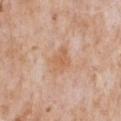Q: Was a biopsy performed?
A: no biopsy performed (imaged during a skin exam)
Q: Where on the body is the lesion?
A: the chest
Q: What kind of image is this?
A: total-body-photography crop, ~15 mm field of view
Q: What are the patient's age and sex?
A: male, roughly 65 years of age
Q: What lighting was used for the tile?
A: white-light
Q: Automated lesion metrics?
A: a border-irregularity index near 3/10, internal color variation of about 2 on a 0–10 scale, and a peripheral color-asymmetry measure near 0.5; a classifier nevus-likeness of about 0/100